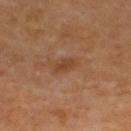Clinical impression:
Part of a total-body skin-imaging series; this lesion was reviewed on a skin check and was not flagged for biopsy.
Context:
A male subject aged approximately 70. From the upper back. Cropped from a whole-body photographic skin survey; the tile spans about 15 mm.